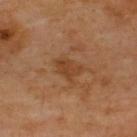Q: Is there a histopathology result?
A: imaged on a skin check; not biopsied
Q: Who is the patient?
A: aged approximately 65
Q: What kind of image is this?
A: ~15 mm crop, total-body skin-cancer survey
Q: How was the tile lit?
A: cross-polarized illumination
Q: What is the lesion's diameter?
A: ~3 mm (longest diameter)
Q: Automated lesion metrics?
A: a shape eccentricity near 0.65 and a symmetry-axis asymmetry near 0.3; an average lesion color of about L≈41 a*≈22 b*≈35 (CIELAB), about 7 CIELAB-L* units darker than the surrounding skin, and a normalized lesion–skin contrast near 6.5; a classifier nevus-likeness of about 0/100 and lesion-presence confidence of about 100/100
Q: Where on the body is the lesion?
A: the upper back